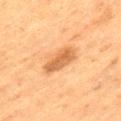- notes — total-body-photography surveillance lesion; no biopsy
- lesion size — ~4.5 mm (longest diameter)
- subject — male, aged 73 to 77
- image source — total-body-photography crop, ~15 mm field of view
- automated metrics — an average lesion color of about L≈51 a*≈21 b*≈37 (CIELAB) and about 10 CIELAB-L* units darker than the surrounding skin
- lighting — cross-polarized
- body site — the left thigh